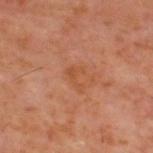Impression:
Imaged during a routine full-body skin examination; the lesion was not biopsied and no histopathology is available.
Acquisition and patient details:
The patient is a male approximately 60 years of age. A 15 mm close-up extracted from a 3D total-body photography capture. Located on the upper back.